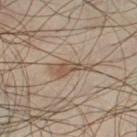image-analysis metrics: a lesion area of about 3.5 mm², an eccentricity of roughly 0.9, and a shape-asymmetry score of about 0.4 (0 = symmetric); about 10 CIELAB-L* units darker than the surrounding skin and a normalized border contrast of about 7.5; a classifier nevus-likeness of about 65/100 and a lesion-detection confidence of about 90/100 | lesion size: ~3 mm (longest diameter) | site: the left thigh | acquisition: ~15 mm crop, total-body skin-cancer survey | illumination: cross-polarized illumination | subject: male, aged 38 to 42.